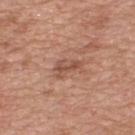| field | value |
|---|---|
| workup | total-body-photography surveillance lesion; no biopsy |
| illumination | white-light |
| acquisition | ~15 mm crop, total-body skin-cancer survey |
| lesion diameter | ≈3 mm |
| patient | male, aged 48 to 52 |
| body site | the back |